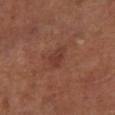Recorded during total-body skin imaging; not selected for excision or biopsy.
A 15 mm close-up tile from a total-body photography series done for melanoma screening.
About 2.5 mm across.
Located on the leg.
This is a cross-polarized tile.
Automated image analysis of the tile measured border irregularity of about 3 on a 0–10 scale, a within-lesion color-variation index near 2/10, and peripheral color asymmetry of about 1.
A male patient, in their mid-60s.